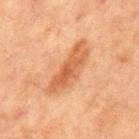| field | value |
|---|---|
| biopsy status | imaged on a skin check; not biopsied |
| image source | 15 mm crop, total-body photography |
| site | the front of the torso |
| automated metrics | a lesion color around L≈49 a*≈22 b*≈34 in CIELAB, a lesion–skin lightness drop of about 9, and a lesion-to-skin contrast of about 7 (normalized; higher = more distinct); a border-irregularity rating of about 4/10, internal color variation of about 4 on a 0–10 scale, and peripheral color asymmetry of about 1; a lesion-detection confidence of about 100/100 |
| patient | male, approximately 65 years of age |
| lesion size | ≈7.5 mm |